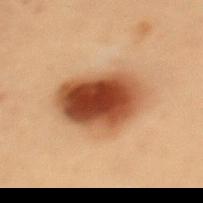{"image": {"source": "total-body photography crop", "field_of_view_mm": 15}, "patient": {"sex": "female", "age_approx": 40}, "site": "mid back"}